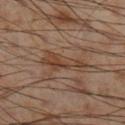Impression:
Imaged during a routine full-body skin examination; the lesion was not biopsied and no histopathology is available.
Image and clinical context:
Imaged with cross-polarized lighting. The lesion is on the right lower leg. A region of skin cropped from a whole-body photographic capture, roughly 15 mm wide. The patient is a male aged 53–57. The recorded lesion diameter is about 5.5 mm.